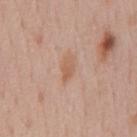Impression:
Part of a total-body skin-imaging series; this lesion was reviewed on a skin check and was not flagged for biopsy.
Image and clinical context:
A male patient aged 53–57. Captured under white-light illumination. On the chest. A lesion tile, about 15 mm wide, cut from a 3D total-body photograph. About 3 mm across.